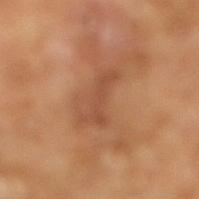Assessment:
Imaged during a routine full-body skin examination; the lesion was not biopsied and no histopathology is available.
Context:
A male subject, aged approximately 65. This image is a 15 mm lesion crop taken from a total-body photograph. The tile uses cross-polarized illumination. Measured at roughly 5 mm in maximum diameter.